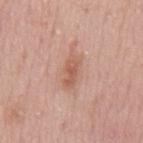Case summary:
* follow-up — catalogued during a skin exam; not biopsied
* subject — male, in their mid- to late 50s
* lesion diameter — ~4 mm (longest diameter)
* image source — total-body-photography crop, ~15 mm field of view
* anatomic site — the mid back